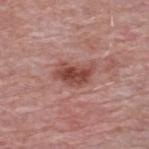Findings:
* biopsy status · catalogued during a skin exam; not biopsied
* lesion diameter · ~4.5 mm (longest diameter)
* anatomic site · the upper back
* imaging modality · 15 mm crop, total-body photography
* patient · male, aged around 65
* lighting · white-light illumination